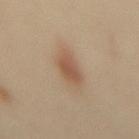Q: Was a biopsy performed?
A: catalogued during a skin exam; not biopsied
Q: How was this image acquired?
A: 15 mm crop, total-body photography
Q: Who is the patient?
A: female, aged around 40
Q: Lesion size?
A: ~3.5 mm (longest diameter)
Q: Lesion location?
A: the mid back
Q: Illumination type?
A: cross-polarized illumination